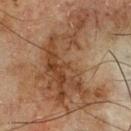workup: no biopsy performed (imaged during a skin exam)
anatomic site: the chest
lesion size: ≈12 mm
automated lesion analysis: an average lesion color of about L≈36 a*≈16 b*≈27 (CIELAB), about 9 CIELAB-L* units darker than the surrounding skin, and a normalized border contrast of about 8; a color-variation rating of about 6/10 and peripheral color asymmetry of about 2
subject: male, aged 68–72
acquisition: ~15 mm crop, total-body skin-cancer survey